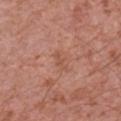This lesion was catalogued during total-body skin photography and was not selected for biopsy.
Captured under white-light illumination.
Automated image analysis of the tile measured a lesion color around L≈53 a*≈24 b*≈30 in CIELAB, about 6 CIELAB-L* units darker than the surrounding skin, and a lesion-to-skin contrast of about 4.5 (normalized; higher = more distinct). And it measured a border-irregularity rating of about 4.5/10, internal color variation of about 1.5 on a 0–10 scale, and peripheral color asymmetry of about 0.5.
A close-up tile cropped from a whole-body skin photograph, about 15 mm across.
The lesion's longest dimension is about 2.5 mm.
The subject is a male about 50 years old.
The lesion is located on the right upper arm.